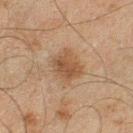Assessment:
This lesion was catalogued during total-body skin photography and was not selected for biopsy.
Acquisition and patient details:
The patient is a male in their mid-40s. On the left lower leg. A 15 mm close-up tile from a total-body photography series done for melanoma screening.